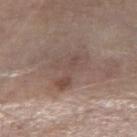workup — total-body-photography surveillance lesion; no biopsy
lesion size — about 5 mm
patient — male, aged 68 to 72
illumination — white-light
acquisition — total-body-photography crop, ~15 mm field of view
site — the right forearm
TBP lesion metrics — a lesion color around L≈48 a*≈16 b*≈22 in CIELAB and a normalized lesion–skin contrast near 5.5; a nevus-likeness score of about 10/100 and a lesion-detection confidence of about 100/100